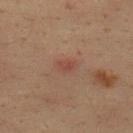Clinical impression: Imaged during a routine full-body skin examination; the lesion was not biopsied and no histopathology is available. Acquisition and patient details: About 2.5 mm across. An algorithmic analysis of the crop reported border irregularity of about 1.5 on a 0–10 scale, a color-variation rating of about 2.5/10, and a peripheral color-asymmetry measure near 1. The software also gave a nevus-likeness score of about 15/100 and a lesion-detection confidence of about 100/100. The lesion is located on the upper back. Cropped from a whole-body photographic skin survey; the tile spans about 15 mm. A male patient roughly 35 years of age.